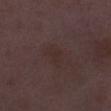Findings:
- biopsy status · imaged on a skin check; not biopsied
- image-analysis metrics · an area of roughly 4.5 mm², an outline eccentricity of about 0.8 (0 = round, 1 = elongated), and a shape-asymmetry score of about 0.35 (0 = symmetric); a border-irregularity rating of about 3.5/10, a color-variation rating of about 1/10, and a peripheral color-asymmetry measure near 0.5; a nevus-likeness score of about 0/100
- tile lighting · white-light
- patient · female, approximately 30 years of age
- anatomic site · the left thigh
- image source · ~15 mm tile from a whole-body skin photo
- lesion diameter · ~3 mm (longest diameter)